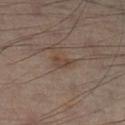Captured during whole-body skin photography for melanoma surveillance; the lesion was not biopsied. A male subject aged around 50. Located on the leg. Automated tile analysis of the lesion measured a detector confidence of about 100 out of 100 that the crop contains a lesion. Cropped from a whole-body photographic skin survey; the tile spans about 15 mm. The tile uses cross-polarized illumination.